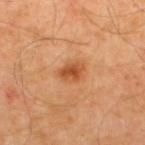The lesion was tiled from a total-body skin photograph and was not biopsied.